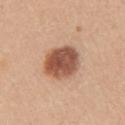site: right upper arm
lighting: white-light
patient:
  sex: female
  age_approx: 45
image:
  source: total-body photography crop
  field_of_view_mm: 15
automated_metrics:
  eccentricity: 0.5
  shape_asymmetry: 0.15
  cielab_L: 53
  cielab_a: 23
  cielab_b: 31
  vs_skin_darker_L: 17.0
  vs_skin_contrast_norm: 10.5
  nevus_likeness_0_100: 95
  lesion_detection_confidence_0_100: 100
lesion_size:
  long_diameter_mm_approx: 4.5